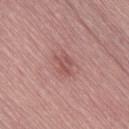workup = no biopsy performed (imaged during a skin exam); site = the right thigh; size = about 3 mm; subject = female, in their mid-60s; lighting = white-light; acquisition = ~15 mm crop, total-body skin-cancer survey.